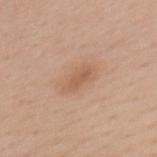This lesion was catalogued during total-body skin photography and was not selected for biopsy.
The lesion is located on the back.
A female patient, aged 38 to 42.
The lesion-visualizer software estimated a footprint of about 8 mm², an outline eccentricity of about 0.85 (0 = round, 1 = elongated), and a symmetry-axis asymmetry near 0.2. The analysis additionally found a lesion color around L≈60 a*≈19 b*≈33 in CIELAB, roughly 7 lightness units darker than nearby skin, and a normalized lesion–skin contrast near 5.5. The analysis additionally found a lesion-detection confidence of about 100/100.
Imaged with white-light lighting.
A roughly 15 mm field-of-view crop from a total-body skin photograph.
Approximately 4.5 mm at its widest.